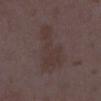<record>
<biopsy_status>not biopsied; imaged during a skin examination</biopsy_status>
<image>
  <source>total-body photography crop</source>
  <field_of_view_mm>15</field_of_view_mm>
</image>
<patient>
  <sex>female</sex>
  <age_approx>35</age_approx>
</patient>
<lesion_size>
  <long_diameter_mm_approx>7.0</long_diameter_mm_approx>
</lesion_size>
<site>right lower leg</site>
</record>